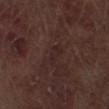Notes:
- follow-up: imaged on a skin check; not biopsied
- subject: male, approximately 70 years of age
- illumination: white-light
- location: the right thigh
- imaging modality: 15 mm crop, total-body photography